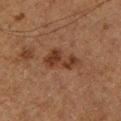notes: total-body-photography surveillance lesion; no biopsy | image: 15 mm crop, total-body photography | lesion diameter: ≈4.5 mm | lighting: cross-polarized | patient: male, about 75 years old | location: the left lower leg.